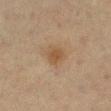No biopsy was performed on this lesion — it was imaged during a full skin examination and was not determined to be concerning.
Approximately 2.5 mm at its widest.
The subject is a female aged approximately 55.
The lesion is on the left lower leg.
A 15 mm close-up extracted from a 3D total-body photography capture.